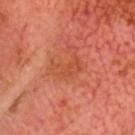Imaged during a routine full-body skin examination; the lesion was not biopsied and no histopathology is available. A male patient, aged 63 to 67. Captured under cross-polarized illumination. About 3.5 mm across. A close-up tile cropped from a whole-body skin photograph, about 15 mm across.